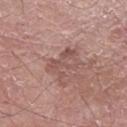Notes:
– notes: catalogued during a skin exam; not biopsied
– subject: male, in their 60s
– imaging modality: 15 mm crop, total-body photography
– image-analysis metrics: a mean CIELAB color near L≈52 a*≈20 b*≈23, roughly 7 lightness units darker than nearby skin, and a normalized border contrast of about 5.5; border irregularity of about 7 on a 0–10 scale, internal color variation of about 4 on a 0–10 scale, and peripheral color asymmetry of about 1; a lesion-detection confidence of about 100/100
– lesion size: ~4.5 mm (longest diameter)
– anatomic site: the right forearm
– tile lighting: white-light